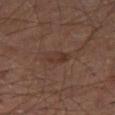notes: total-body-photography surveillance lesion; no biopsy | patient: male, in their mid- to late 50s | imaging modality: ~15 mm crop, total-body skin-cancer survey | image-analysis metrics: a lesion area of about 4.5 mm², a shape eccentricity near 0.8, and a shape-asymmetry score of about 0.2 (0 = symmetric); an average lesion color of about L≈31 a*≈17 b*≈22 (CIELAB), a lesion–skin lightness drop of about 5, and a normalized border contrast of about 5.5 | diameter: ~3 mm (longest diameter) | tile lighting: cross-polarized | site: the right thigh.